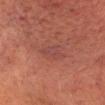Context:
This is a cross-polarized tile. From the head or neck. A male patient approximately 50 years of age. Cropped from a whole-body photographic skin survey; the tile spans about 15 mm. Automated image analysis of the tile measured a border-irregularity index near 3.5/10, internal color variation of about 1 on a 0–10 scale, and radial color variation of about 0.5.